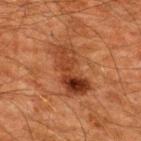Q: Was a biopsy performed?
A: catalogued during a skin exam; not biopsied
Q: What kind of image is this?
A: 15 mm crop, total-body photography
Q: Who is the patient?
A: male, about 60 years old
Q: Lesion location?
A: the upper back